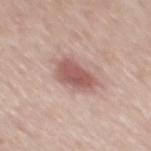The lesion was tiled from a total-body skin photograph and was not biopsied.
Automated tile analysis of the lesion measured a nevus-likeness score of about 90/100.
The tile uses white-light illumination.
Measured at roughly 4.5 mm in maximum diameter.
On the mid back.
A male subject, aged 53 to 57.
This image is a 15 mm lesion crop taken from a total-body photograph.